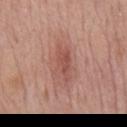follow-up = catalogued during a skin exam; not biopsied